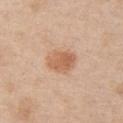follow-up — total-body-photography surveillance lesion; no biopsy
anatomic site — the chest
patient — female, in their mid- to late 40s
acquisition — 15 mm crop, total-body photography
lesion diameter — ~3.5 mm (longest diameter)